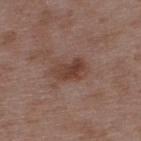follow-up: catalogued during a skin exam; not biopsied
site: the upper back
image source: ~15 mm tile from a whole-body skin photo
lesion size: ≈4 mm
tile lighting: white-light illumination
image-analysis metrics: an area of roughly 7.5 mm², an eccentricity of roughly 0.85, and a shape-asymmetry score of about 0.15 (0 = symmetric); a mean CIELAB color near L≈41 a*≈20 b*≈25, about 9 CIELAB-L* units darker than the surrounding skin, and a lesion-to-skin contrast of about 7.5 (normalized; higher = more distinct); a lesion-detection confidence of about 100/100
patient: male, about 50 years old